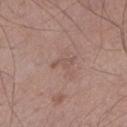Imaged during a routine full-body skin examination; the lesion was not biopsied and no histopathology is available.
A male subject, aged approximately 60.
The lesion is located on the leg.
This image is a 15 mm lesion crop taken from a total-body photograph.